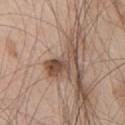notes — catalogued during a skin exam; not biopsied
imaging modality — ~15 mm tile from a whole-body skin photo
subject — male, in their mid- to late 60s
location — the chest